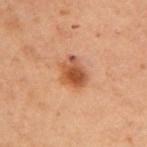<case>
  <biopsy_status>not biopsied; imaged during a skin examination</biopsy_status>
  <lesion_size>
    <long_diameter_mm_approx>3.5</long_diameter_mm_approx>
  </lesion_size>
  <lighting>cross-polarized</lighting>
  <image>
    <source>total-body photography crop</source>
    <field_of_view_mm>15</field_of_view_mm>
  </image>
  <patient>
    <sex>female</sex>
    <age_approx>55</age_approx>
  </patient>
  <site>left upper arm</site>
</case>